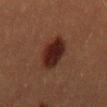Imaged during a routine full-body skin examination; the lesion was not biopsied and no histopathology is available. The lesion is located on the back. A 15 mm crop from a total-body photograph taken for skin-cancer surveillance. A male patient, roughly 40 years of age.